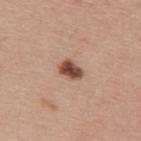Clinical summary:
Cropped from a total-body skin-imaging series; the visible field is about 15 mm. Located on the back. About 3 mm across. A male patient, approximately 40 years of age. The tile uses white-light illumination.